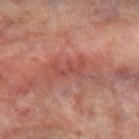Q: Is there a histopathology result?
A: catalogued during a skin exam; not biopsied
Q: What is the lesion's diameter?
A: ≈3.5 mm
Q: What are the patient's age and sex?
A: female, aged 63–67
Q: How was this image acquired?
A: total-body-photography crop, ~15 mm field of view
Q: Illumination type?
A: cross-polarized
Q: Lesion location?
A: the left forearm